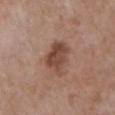Q: Is there a histopathology result?
A: catalogued during a skin exam; not biopsied
Q: How was this image acquired?
A: ~15 mm crop, total-body skin-cancer survey
Q: Lesion location?
A: the chest
Q: Who is the patient?
A: male, about 85 years old or older